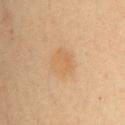{
  "biopsy_status": "not biopsied; imaged during a skin examination",
  "image": {
    "source": "total-body photography crop",
    "field_of_view_mm": 15
  },
  "lighting": "cross-polarized",
  "patient": {
    "sex": "female",
    "age_approx": 30
  },
  "site": "arm",
  "lesion_size": {
    "long_diameter_mm_approx": 3.0
  }
}